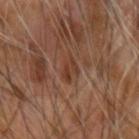Recorded during total-body skin imaging; not selected for excision or biopsy.